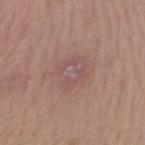Clinical summary: Imaged with white-light lighting. Cropped from a whole-body photographic skin survey; the tile spans about 15 mm. The lesion is on the right forearm. Approximately 3.5 mm at its widest. The patient is a female aged approximately 45.